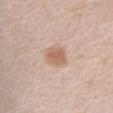<tbp_lesion>
<biopsy_status>not biopsied; imaged during a skin examination</biopsy_status>
<lesion_size>
  <long_diameter_mm_approx>3.0</long_diameter_mm_approx>
</lesion_size>
<image>
  <source>total-body photography crop</source>
  <field_of_view_mm>15</field_of_view_mm>
</image>
<patient>
  <sex>male</sex>
  <age_approx>80</age_approx>
</patient>
<site>front of the torso</site>
</tbp_lesion>